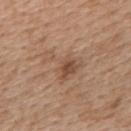  biopsy_status: not biopsied; imaged during a skin examination
  image:
    source: total-body photography crop
    field_of_view_mm: 15
  site: upper back
  patient:
    sex: female
    age_approx: 55
  lesion_size:
    long_diameter_mm_approx: 5.5
  lighting: white-light